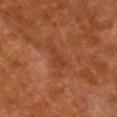biopsy status — total-body-photography surveillance lesion; no biopsy
acquisition — 15 mm crop, total-body photography
diameter — about 3 mm
patient — female, aged approximately 50
anatomic site — the chest
automated metrics — a footprint of about 2.5 mm², an eccentricity of roughly 0.9, and two-axis asymmetry of about 0.5; a mean CIELAB color near L≈32 a*≈24 b*≈29, about 5 CIELAB-L* units darker than the surrounding skin, and a normalized border contrast of about 4.5
tile lighting — cross-polarized illumination